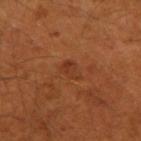patient=male, about 50 years old | image=15 mm crop, total-body photography | tile lighting=cross-polarized | lesion size=≈3 mm | site=the arm.